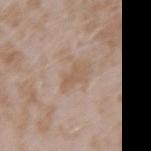{
  "biopsy_status": "not biopsied; imaged during a skin examination",
  "patient": {
    "sex": "female",
    "age_approx": 25
  },
  "site": "left forearm",
  "lesion_size": {
    "long_diameter_mm_approx": 3.0
  },
  "lighting": "white-light",
  "image": {
    "source": "total-body photography crop",
    "field_of_view_mm": 15
  }
}